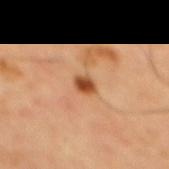{
  "biopsy_status": "not biopsied; imaged during a skin examination",
  "patient": {
    "sex": "male",
    "age_approx": 65
  },
  "site": "mid back",
  "image": {
    "source": "total-body photography crop",
    "field_of_view_mm": 15
  }
}